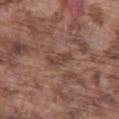This lesion was catalogued during total-body skin photography and was not selected for biopsy.
A male subject, approximately 75 years of age.
On the left thigh.
A lesion tile, about 15 mm wide, cut from a 3D total-body photograph.